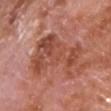Background: Located on the front of the torso. A lesion tile, about 15 mm wide, cut from a 3D total-body photograph. The patient is a male aged 63–67.